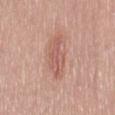workup = total-body-photography surveillance lesion; no biopsy
anatomic site = the right thigh
lighting = white-light
imaging modality = 15 mm crop, total-body photography
subject = female, approximately 70 years of age
lesion diameter = ≈5.5 mm